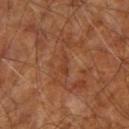The patient is aged around 65. A roughly 15 mm field-of-view crop from a total-body skin photograph. The lesion-visualizer software estimated an average lesion color of about L≈39 a*≈25 b*≈33 (CIELAB). And it measured border irregularity of about 4.5 on a 0–10 scale and a within-lesion color-variation index near 0/10. The software also gave an automated nevus-likeness rating near 0 out of 100 and a lesion-detection confidence of about 65/100. Located on the right upper arm. The lesion's longest dimension is about 2.5 mm.